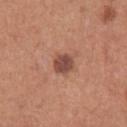No biopsy was performed on this lesion — it was imaged during a full skin examination and was not determined to be concerning. This is a white-light tile. The lesion is located on the right upper arm. The patient is a female roughly 30 years of age. A region of skin cropped from a whole-body photographic capture, roughly 15 mm wide.